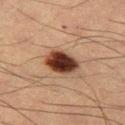Recorded during total-body skin imaging; not selected for excision or biopsy.
The lesion is on the right thigh.
A male patient roughly 55 years of age.
About 4.5 mm across.
A region of skin cropped from a whole-body photographic capture, roughly 15 mm wide.
The tile uses cross-polarized illumination.
The lesion-visualizer software estimated a lesion area of about 10 mm² and a symmetry-axis asymmetry near 0.2. The analysis additionally found border irregularity of about 1.5 on a 0–10 scale, a color-variation rating of about 7/10, and peripheral color asymmetry of about 2.